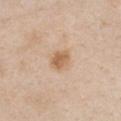workup: catalogued during a skin exam; not biopsied | image source: 15 mm crop, total-body photography | subject: female, in their 40s | size: about 2.5 mm | illumination: white-light illumination | site: the chest.